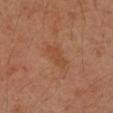The lesion was photographed on a routine skin check and not biopsied; there is no pathology result.
The lesion-visualizer software estimated a footprint of about 3.5 mm², a shape eccentricity near 0.9, and a symmetry-axis asymmetry near 0.35. And it measured a mean CIELAB color near L≈47 a*≈24 b*≈35 and roughly 6 lightness units darker than nearby skin. The software also gave lesion-presence confidence of about 100/100.
The lesion's longest dimension is about 3 mm.
The lesion is on the arm.
A 15 mm close-up extracted from a 3D total-body photography capture.
The patient is a female aged 38 to 42.